The lesion is on the chest · this is a white-light tile · The total-body-photography lesion software estimated a mean CIELAB color near L≈48 a*≈24 b*≈20, roughly 10 lightness units darker than nearby skin, and a normalized border contrast of about 8. The analysis additionally found a border-irregularity index near 5/10 and peripheral color asymmetry of about 2. The software also gave a nevus-likeness score of about 20/100 and lesion-presence confidence of about 100/100 · the recorded lesion diameter is about 9.5 mm · a region of skin cropped from a whole-body photographic capture, roughly 15 mm wide · the subject is a male aged approximately 55: 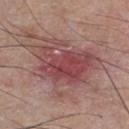Histopathological examination showed a malignant skin lesion: nodular basal cell carcinoma.